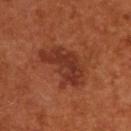• notes: no biopsy performed (imaged during a skin exam)
• lesion diameter: about 6 mm
• location: the upper back
• patient: male, aged around 55
• image-analysis metrics: a mean CIELAB color near L≈32 a*≈25 b*≈29 and a normalized lesion–skin contrast near 7.5; a border-irregularity rating of about 5/10 and a color-variation rating of about 3/10
• image: total-body-photography crop, ~15 mm field of view